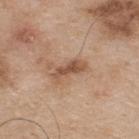Findings:
– follow-up · imaged on a skin check; not biopsied
– illumination · white-light
– automated lesion analysis · an automated nevus-likeness rating near 10 out of 100 and a detector confidence of about 100 out of 100 that the crop contains a lesion
– lesion size · ≈4.5 mm
– patient · male, about 75 years old
– anatomic site · the back
– acquisition · total-body-photography crop, ~15 mm field of view